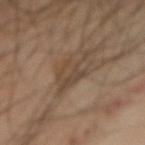biopsy_status: not biopsied; imaged during a skin examination
image:
  source: total-body photography crop
  field_of_view_mm: 15
lesion_size:
  long_diameter_mm_approx: 5.0
patient:
  sex: male
  age_approx: 40
automated_metrics:
  area_mm2_approx: 9.0
  eccentricity: 0.75
  shape_asymmetry: 0.45
  cielab_L: 42
  cielab_a: 14
  cielab_b: 26
  border_irregularity_0_10: 6.5
  color_variation_0_10: 4.0
  nevus_likeness_0_100: 5
  lesion_detection_confidence_0_100: 60
lighting: cross-polarized
site: right forearm